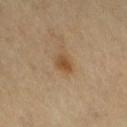No biopsy was performed on this lesion — it was imaged during a full skin examination and was not determined to be concerning.
A female subject aged 68 to 72.
Imaged with cross-polarized lighting.
The lesion's longest dimension is about 2.5 mm.
Automated image analysis of the tile measured an area of roughly 4 mm², an outline eccentricity of about 0.65 (0 = round, 1 = elongated), and two-axis asymmetry of about 0.3. The analysis additionally found a border-irregularity rating of about 2.5/10, a within-lesion color-variation index near 3/10, and peripheral color asymmetry of about 1. The software also gave a nevus-likeness score of about 80/100 and a detector confidence of about 100 out of 100 that the crop contains a lesion.
The lesion is on the right thigh.
A 15 mm close-up tile from a total-body photography series done for melanoma screening.